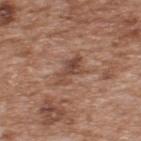Clinical impression: No biopsy was performed on this lesion — it was imaged during a full skin examination and was not determined to be concerning. Image and clinical context: The patient is a male aged around 65. A 15 mm close-up tile from a total-body photography series done for melanoma screening. From the upper back. Measured at roughly 4 mm in maximum diameter. The total-body-photography lesion software estimated a footprint of about 6 mm² and a symmetry-axis asymmetry near 0.45. And it measured a classifier nevus-likeness of about 0/100. This is a white-light tile.